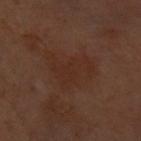Case summary:
– biopsy status: no biopsy performed (imaged during a skin exam)
– image: ~15 mm crop, total-body skin-cancer survey
– subject: female, aged 58 to 62
– image-analysis metrics: an area of roughly 14 mm² and a shape-asymmetry score of about 0.45 (0 = symmetric); about 3 CIELAB-L* units darker than the surrounding skin and a normalized lesion–skin contrast near 4.5; a border-irregularity rating of about 5/10, internal color variation of about 2 on a 0–10 scale, and peripheral color asymmetry of about 0.5; a nevus-likeness score of about 0/100
– location: the front of the torso